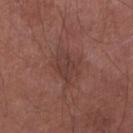<record>
<biopsy_status>not biopsied; imaged during a skin examination</biopsy_status>
<patient>
  <sex>male</sex>
  <age_approx>55</age_approx>
</patient>
<image>
  <source>total-body photography crop</source>
  <field_of_view_mm>15</field_of_view_mm>
</image>
<site>right lower leg</site>
<lighting>white-light</lighting>
</record>